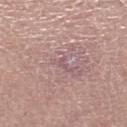workup: total-body-photography surveillance lesion; no biopsy | automated metrics: a lesion area of about 1 mm² and two-axis asymmetry of about 0.6; a mean CIELAB color near L≈54 a*≈21 b*≈17, about 6 CIELAB-L* units darker than the surrounding skin, and a normalized border contrast of about 4.5; a lesion-detection confidence of about 45/100 | patient: female, in their 60s | lesion diameter: ≈1.5 mm | location: the right lower leg | imaging modality: total-body-photography crop, ~15 mm field of view | tile lighting: white-light.